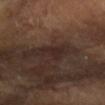biopsy_status: not biopsied; imaged during a skin examination
lesion_size:
  long_diameter_mm_approx: 3.0
lighting: cross-polarized
automated_metrics:
  vs_skin_darker_L: 5.0
  vs_skin_contrast_norm: 6.0
  border_irregularity_0_10: 6.0
  color_variation_0_10: 2.0
  peripheral_color_asymmetry: 0.5
image:
  source: total-body photography crop
  field_of_view_mm: 15
patient:
  age_approx: 60
site: right forearm